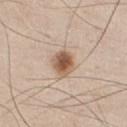<tbp_lesion>
  <biopsy_status>not biopsied; imaged during a skin examination</biopsy_status>
  <lighting>white-light</lighting>
  <site>chest</site>
  <patient>
    <sex>male</sex>
    <age_approx>45</age_approx>
  </patient>
  <lesion_size>
    <long_diameter_mm_approx>3.5</long_diameter_mm_approx>
  </lesion_size>
  <image>
    <source>total-body photography crop</source>
    <field_of_view_mm>15</field_of_view_mm>
  </image>
  <automated_metrics>
    <eccentricity>0.6</eccentricity>
    <shape_asymmetry>0.2</shape_asymmetry>
    <nevus_likeness_0_100>100</nevus_likeness_0_100>
    <lesion_detection_confidence_0_100>100</lesion_detection_confidence_0_100>
  </automated_metrics>
</tbp_lesion>